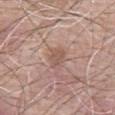No biopsy was performed on this lesion — it was imaged during a full skin examination and was not determined to be concerning. Captured under white-light illumination. From the abdomen. A male patient aged around 60. A lesion tile, about 15 mm wide, cut from a 3D total-body photograph. Longest diameter approximately 2.5 mm. The total-body-photography lesion software estimated an average lesion color of about L≈54 a*≈19 b*≈25 (CIELAB), about 7 CIELAB-L* units darker than the surrounding skin, and a normalized lesion–skin contrast near 5.5. And it measured a border-irregularity index near 2/10 and a within-lesion color-variation index near 1.5/10.